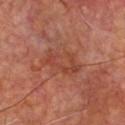Assessment: No biopsy was performed on this lesion — it was imaged during a full skin examination and was not determined to be concerning. Clinical summary: This is a cross-polarized tile. The lesion's longest dimension is about 5 mm. Located on the chest. A male subject, aged around 60. A 15 mm close-up extracted from a 3D total-body photography capture.